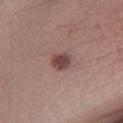  biopsy_status: not biopsied; imaged during a skin examination
  lesion_size:
    long_diameter_mm_approx: 2.5
  image:
    source: total-body photography crop
    field_of_view_mm: 15
  site: leg
  lighting: white-light
  patient:
    sex: male
    age_approx: 45